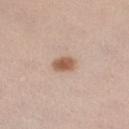Part of a total-body skin-imaging series; this lesion was reviewed on a skin check and was not flagged for biopsy. The lesion's longest dimension is about 3 mm. The tile uses white-light illumination. A female subject, aged 38–42. From the right lower leg. A 15 mm close-up extracted from a 3D total-body photography capture.